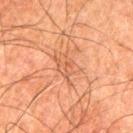No biopsy was performed on this lesion — it was imaged during a full skin examination and was not determined to be concerning. Captured under cross-polarized illumination. Cropped from a whole-body photographic skin survey; the tile spans about 15 mm. A male subject aged 78 to 82. Located on the left thigh.